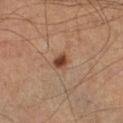<case>
  <biopsy_status>not biopsied; imaged during a skin examination</biopsy_status>
  <site>right lower leg</site>
  <lighting>cross-polarized</lighting>
  <image>
    <source>total-body photography crop</source>
    <field_of_view_mm>15</field_of_view_mm>
  </image>
  <patient>
    <sex>male</sex>
    <age_approx>65</age_approx>
  </patient>
  <lesion_size>
    <long_diameter_mm_approx>2.5</long_diameter_mm_approx>
  </lesion_size>
  <automated_metrics>
    <cielab_L>42</cielab_L>
    <cielab_a>20</cielab_a>
    <cielab_b>30</cielab_b>
    <vs_skin_darker_L>13.0</vs_skin_darker_L>
    <vs_skin_contrast_norm>10.0</vs_skin_contrast_norm>
    <border_irregularity_0_10>2.5</border_irregularity_0_10>
    <color_variation_0_10>2.0</color_variation_0_10>
    <peripheral_color_asymmetry>0.5</peripheral_color_asymmetry>
  </automated_metrics>
</case>